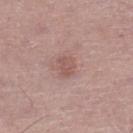Findings:
- anatomic site: the left lower leg
- subject: male, roughly 65 years of age
- image: ~15 mm crop, total-body skin-cancer survey